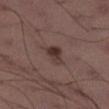Q: Was a biopsy performed?
A: total-body-photography surveillance lesion; no biopsy
Q: What is the lesion's diameter?
A: about 2.5 mm
Q: How was this image acquired?
A: ~15 mm tile from a whole-body skin photo
Q: What is the anatomic site?
A: the right lower leg
Q: What are the patient's age and sex?
A: male, aged approximately 40
Q: Illumination type?
A: white-light illumination